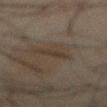patient:
  sex: male
  age_approx: 45
image:
  source: total-body photography crop
  field_of_view_mm: 15
lighting: cross-polarized
site: front of the torso
lesion_size:
  long_diameter_mm_approx: 3.5
automated_metrics:
  area_mm2_approx: 4.0
  eccentricity: 0.95
  shape_asymmetry: 0.3